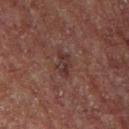Q: How large is the lesion?
A: ≈3.5 mm
Q: Who is the patient?
A: male, aged approximately 55
Q: What is the anatomic site?
A: the front of the torso
Q: How was the tile lit?
A: cross-polarized illumination
Q: What kind of image is this?
A: ~15 mm crop, total-body skin-cancer survey